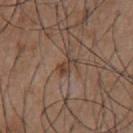A 15 mm close-up tile from a total-body photography series done for melanoma screening. On the chest. The patient is a male aged around 55.